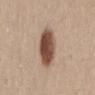No biopsy was performed on this lesion — it was imaged during a full skin examination and was not determined to be concerning. A close-up tile cropped from a whole-body skin photograph, about 15 mm across. The lesion is located on the mid back. The total-body-photography lesion software estimated a footprint of about 14 mm² and an outline eccentricity of about 0.75 (0 = round, 1 = elongated). The software also gave a mean CIELAB color near L≈49 a*≈18 b*≈27, a lesion–skin lightness drop of about 18, and a lesion-to-skin contrast of about 12 (normalized; higher = more distinct). It also reported a classifier nevus-likeness of about 100/100 and a lesion-detection confidence of about 100/100. A female patient, in their 30s.